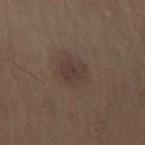biopsy status: total-body-photography surveillance lesion; no biopsy | imaging modality: ~15 mm tile from a whole-body skin photo | TBP lesion metrics: a lesion color around L≈31 a*≈12 b*≈18 in CIELAB and about 5 CIELAB-L* units darker than the surrounding skin; a border-irregularity index near 2.5/10, a within-lesion color-variation index near 3/10, and peripheral color asymmetry of about 1 | body site: the right thigh | tile lighting: cross-polarized | subject: female, aged approximately 50.